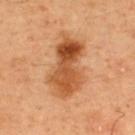notes — imaged on a skin check; not biopsied
image-analysis metrics — a shape eccentricity near 0.85 and two-axis asymmetry of about 0.25; an average lesion color of about L≈51 a*≈25 b*≈39 (CIELAB) and roughly 13 lightness units darker than nearby skin; a border-irregularity rating of about 3.5/10, internal color variation of about 9 on a 0–10 scale, and peripheral color asymmetry of about 3; a lesion-detection confidence of about 100/100
location — the upper back
subject — male, in their 50s
image source — ~15 mm crop, total-body skin-cancer survey